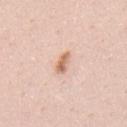Clinical summary: A 15 mm close-up tile from a total-body photography series done for melanoma screening. From the upper back. The total-body-photography lesion software estimated a border-irregularity index near 2.5/10, a within-lesion color-variation index near 3/10, and radial color variation of about 1. It also reported a classifier nevus-likeness of about 95/100 and a detector confidence of about 100 out of 100 that the crop contains a lesion. Longest diameter approximately 2.5 mm. The tile uses white-light illumination. The patient is a male approximately 25 years of age.